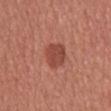Imaged during a routine full-body skin examination; the lesion was not biopsied and no histopathology is available. Measured at roughly 3.5 mm in maximum diameter. This is a white-light tile. The lesion is located on the abdomen. The subject is a male aged 43–47. The total-body-photography lesion software estimated a mean CIELAB color near L≈46 a*≈28 b*≈29 and a lesion-to-skin contrast of about 7.5 (normalized; higher = more distinct). A lesion tile, about 15 mm wide, cut from a 3D total-body photograph.